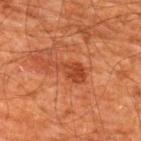Recorded during total-body skin imaging; not selected for excision or biopsy. Cropped from a total-body skin-imaging series; the visible field is about 15 mm. Located on the back. A male patient aged 58 to 62. Imaged with cross-polarized lighting.